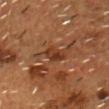Recorded during total-body skin imaging; not selected for excision or biopsy. Imaged with cross-polarized lighting. Located on the head or neck. Approximately 3 mm at its widest. A 15 mm crop from a total-body photograph taken for skin-cancer surveillance. The patient is a male approximately 55 years of age.